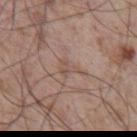Q: Was this lesion biopsied?
A: no biopsy performed (imaged during a skin exam)
Q: What kind of image is this?
A: 15 mm crop, total-body photography
Q: Lesion location?
A: the chest
Q: What are the patient's age and sex?
A: male, approximately 55 years of age
Q: Automated lesion metrics?
A: a lesion color around L≈52 a*≈17 b*≈25 in CIELAB and a normalized lesion–skin contrast near 5; a nevus-likeness score of about 0/100 and a detector confidence of about 60 out of 100 that the crop contains a lesion
Q: Lesion size?
A: ≈2.5 mm
Q: What lighting was used for the tile?
A: white-light illumination